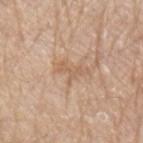{"biopsy_status": "not biopsied; imaged during a skin examination", "lighting": "white-light", "image": {"source": "total-body photography crop", "field_of_view_mm": 15}, "automated_metrics": {"border_irregularity_0_10": 8.0, "color_variation_0_10": 1.0, "peripheral_color_asymmetry": 0.0, "nevus_likeness_0_100": 0, "lesion_detection_confidence_0_100": 95}, "site": "left upper arm", "lesion_size": {"long_diameter_mm_approx": 3.0}, "patient": {"sex": "male", "age_approx": 70}}